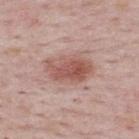| feature | finding |
|---|---|
| biopsy status | no biopsy performed (imaged during a skin exam) |
| subject | female, about 50 years old |
| lighting | white-light illumination |
| image | ~15 mm tile from a whole-body skin photo |
| diameter | ≈5 mm |
| location | the upper back |